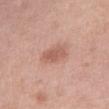biopsy status=imaged on a skin check; not biopsied | lighting=white-light | anatomic site=the leg | lesion size=about 3.5 mm | image source=~15 mm crop, total-body skin-cancer survey | image-analysis metrics=an area of roughly 5.5 mm², an eccentricity of roughly 0.8, and two-axis asymmetry of about 0.25; an average lesion color of about L≈58 a*≈23 b*≈28 (CIELAB), a lesion–skin lightness drop of about 10, and a normalized border contrast of about 6.5; a border-irregularity index near 2.5/10 and a within-lesion color-variation index near 2.5/10 | subject=female, in their 40s.